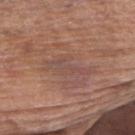A male patient, about 80 years old. The lesion is located on the upper back. Captured under white-light illumination. Automated image analysis of the tile measured a footprint of about 5.5 mm², a shape eccentricity near 0.95, and a symmetry-axis asymmetry near 0.4. The analysis additionally found a mean CIELAB color near L≈48 a*≈19 b*≈23 and a lesion-to-skin contrast of about 5 (normalized; higher = more distinct). It also reported border irregularity of about 6 on a 0–10 scale, internal color variation of about 2 on a 0–10 scale, and a peripheral color-asymmetry measure near 0.5. It also reported an automated nevus-likeness rating near 0 out of 100 and a detector confidence of about 65 out of 100 that the crop contains a lesion. A lesion tile, about 15 mm wide, cut from a 3D total-body photograph. Longest diameter approximately 5 mm.